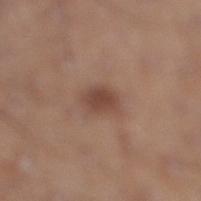The total-body-photography lesion software estimated a footprint of about 5.5 mm² and a shape-asymmetry score of about 0.25 (0 = symmetric). And it measured a mean CIELAB color near L≈45 a*≈19 b*≈26, a lesion–skin lightness drop of about 10, and a normalized border contrast of about 7.5. From the leg. A male patient in their 60s. A lesion tile, about 15 mm wide, cut from a 3D total-body photograph. About 3 mm across.